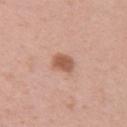Assessment: The lesion was tiled from a total-body skin photograph and was not biopsied. Acquisition and patient details: Measured at roughly 2.5 mm in maximum diameter. The lesion is located on the arm. The tile uses white-light illumination. The subject is a female roughly 40 years of age. An algorithmic analysis of the crop reported a lesion–skin lightness drop of about 12 and a lesion-to-skin contrast of about 8 (normalized; higher = more distinct). The software also gave a classifier nevus-likeness of about 90/100. A region of skin cropped from a whole-body photographic capture, roughly 15 mm wide.